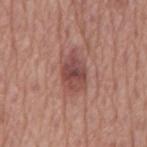Imaged during a routine full-body skin examination; the lesion was not biopsied and no histopathology is available. A lesion tile, about 15 mm wide, cut from a 3D total-body photograph. A male patient, aged 73 to 77. On the mid back. The lesion-visualizer software estimated an area of roughly 11 mm², an eccentricity of roughly 0.8, and a symmetry-axis asymmetry near 0.2. And it measured a mean CIELAB color near L≈48 a*≈23 b*≈23, roughly 11 lightness units darker than nearby skin, and a lesion-to-skin contrast of about 8 (normalized; higher = more distinct). Longest diameter approximately 5 mm. This is a white-light tile.